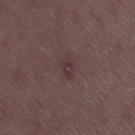notes: total-body-photography surveillance lesion; no biopsy
acquisition: 15 mm crop, total-body photography
lesion diameter: ≈3 mm
patient: male, about 50 years old
lighting: white-light illumination